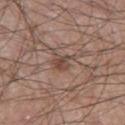size = ≈2.5 mm
subject = male, about 60 years old
site = the left thigh
acquisition = ~15 mm tile from a whole-body skin photo
lighting = white-light
image-analysis metrics = a mean CIELAB color near L≈46 a*≈17 b*≈24, roughly 9 lightness units darker than nearby skin, and a normalized border contrast of about 7; a lesion-detection confidence of about 100/100